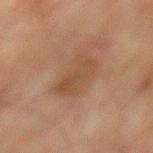Case summary:
- workup — catalogued during a skin exam; not biopsied
- size — about 4.5 mm
- patient — male, roughly 75 years of age
- body site — the mid back
- imaging modality — ~15 mm tile from a whole-body skin photo
- lighting — cross-polarized